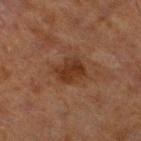Notes:
– biopsy status · no biopsy performed (imaged during a skin exam)
– imaging modality · 15 mm crop, total-body photography
– lesion size · ≈3.5 mm
– subject · male, roughly 70 years of age
– lighting · cross-polarized
– location · the leg